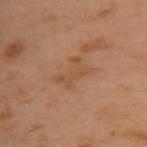{
  "biopsy_status": "not biopsied; imaged during a skin examination",
  "site": "upper back",
  "image": {
    "source": "total-body photography crop",
    "field_of_view_mm": 15
  },
  "automated_metrics": {
    "cielab_L": 41,
    "cielab_a": 18,
    "cielab_b": 29,
    "vs_skin_darker_L": 5.0,
    "vs_skin_contrast_norm": 5.0
  },
  "patient": {
    "sex": "female",
    "age_approx": 55
  }
}